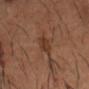Notes:
• follow-up: imaged on a skin check; not biopsied
• site: the head or neck
• patient: male, aged 33 to 37
• image: 15 mm crop, total-body photography